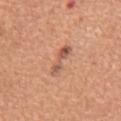workup: imaged on a skin check; not biopsied | lighting: white-light illumination | subject: male, aged approximately 65 | anatomic site: the mid back | image source: ~15 mm tile from a whole-body skin photo | diameter: ≈4 mm | automated lesion analysis: an eccentricity of roughly 0.95 and a shape-asymmetry score of about 0.25 (0 = symmetric).